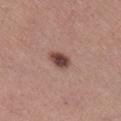Assessment:
The lesion was tiled from a total-body skin photograph and was not biopsied.
Image and clinical context:
Cropped from a whole-body photographic skin survey; the tile spans about 15 mm. Longest diameter approximately 3 mm. A female patient, aged 53 to 57. From the right lower leg. The tile uses white-light illumination. An algorithmic analysis of the crop reported a classifier nevus-likeness of about 95/100 and lesion-presence confidence of about 100/100.